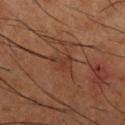Assessment: Imaged during a routine full-body skin examination; the lesion was not biopsied and no histopathology is available. Acquisition and patient details: The lesion-visualizer software estimated an eccentricity of roughly 0.7 and a shape-asymmetry score of about 0.45 (0 = symmetric). The analysis additionally found a border-irregularity index near 4.5/10, a color-variation rating of about 2.5/10, and radial color variation of about 1. The analysis additionally found a lesion-detection confidence of about 70/100. A lesion tile, about 15 mm wide, cut from a 3D total-body photograph. The tile uses cross-polarized illumination. A male subject aged 63 to 67. The lesion's longest dimension is about 3 mm. The lesion is on the right lower leg.